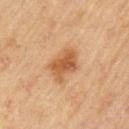Q: Was this lesion biopsied?
A: no biopsy performed (imaged during a skin exam)
Q: Automated lesion metrics?
A: a border-irregularity rating of about 3/10, a within-lesion color-variation index near 4/10, and a peripheral color-asymmetry measure near 1
Q: Who is the patient?
A: male, aged 68–72
Q: Where on the body is the lesion?
A: the left upper arm
Q: What is the imaging modality?
A: ~15 mm tile from a whole-body skin photo